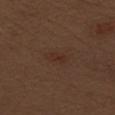This lesion was catalogued during total-body skin photography and was not selected for biopsy.
Longest diameter approximately 3 mm.
Cropped from a total-body skin-imaging series; the visible field is about 15 mm.
A male subject about 70 years old.
The lesion is located on the left upper arm.
An algorithmic analysis of the crop reported an area of roughly 3 mm², an outline eccentricity of about 0.9 (0 = round, 1 = elongated), and two-axis asymmetry of about 0.3. The software also gave a mean CIELAB color near L≈29 a*≈19 b*≈23, a lesion–skin lightness drop of about 4, and a normalized border contrast of about 5.